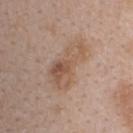Captured during whole-body skin photography for melanoma surveillance; the lesion was not biopsied.
A female subject about 45 years old.
Cropped from a whole-body photographic skin survey; the tile spans about 15 mm.
Measured at roughly 6.5 mm in maximum diameter.
The total-body-photography lesion software estimated border irregularity of about 4.5 on a 0–10 scale. It also reported a classifier nevus-likeness of about 5/100 and lesion-presence confidence of about 100/100.
Located on the upper back.
Captured under white-light illumination.